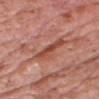This lesion was catalogued during total-body skin photography and was not selected for biopsy. The lesion is located on the head or neck. A 15 mm close-up extracted from a 3D total-body photography capture. A male subject, aged 58–62. The recorded lesion diameter is about 4 mm. Imaged with white-light lighting.